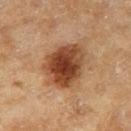Imaged during a routine full-body skin examination; the lesion was not biopsied and no histopathology is available. The lesion is located on the right thigh. A female subject approximately 60 years of age. This is a cross-polarized tile. A region of skin cropped from a whole-body photographic capture, roughly 15 mm wide.